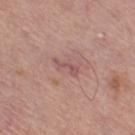The lesion was tiled from a total-body skin photograph and was not biopsied.
Imaged with white-light lighting.
From the leg.
A male subject, roughly 70 years of age.
A 15 mm close-up extracted from a 3D total-body photography capture.
The lesion's longest dimension is about 3 mm.